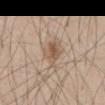Case summary:
• notes · catalogued during a skin exam; not biopsied
• patient · male, roughly 45 years of age
• automated lesion analysis · a color-variation rating of about 5/10 and peripheral color asymmetry of about 1.5
• lesion size · ~3.5 mm (longest diameter)
• body site · the abdomen
• acquisition · 15 mm crop, total-body photography